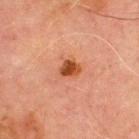Background: From the chest. Cropped from a whole-body photographic skin survey; the tile spans about 15 mm. A male patient, aged around 70. About 2.5 mm across.